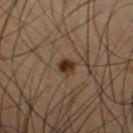Assessment:
The lesion was tiled from a total-body skin photograph and was not biopsied.
Context:
The lesion is located on the right thigh. Longest diameter approximately 2.5 mm. A male subject aged approximately 65. An algorithmic analysis of the crop reported an average lesion color of about L≈31 a*≈16 b*≈26 (CIELAB) and a lesion–skin lightness drop of about 12. The software also gave a detector confidence of about 100 out of 100 that the crop contains a lesion. The tile uses cross-polarized illumination. Cropped from a total-body skin-imaging series; the visible field is about 15 mm.